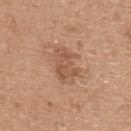<tbp_lesion>
  <biopsy_status>not biopsied; imaged during a skin examination</biopsy_status>
  <site>upper back</site>
  <image>
    <source>total-body photography crop</source>
    <field_of_view_mm>15</field_of_view_mm>
  </image>
  <patient>
    <sex>male</sex>
    <age_approx>40</age_approx>
  </patient>
  <lighting>white-light</lighting>
  <lesion_size>
    <long_diameter_mm_approx>4.5</long_diameter_mm_approx>
  </lesion_size>
</tbp_lesion>